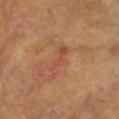• biopsy status — total-body-photography surveillance lesion; no biopsy
• imaging modality — 15 mm crop, total-body photography
• illumination — cross-polarized
• diameter — about 4 mm
• subject — female, about 75 years old
• anatomic site — the left forearm